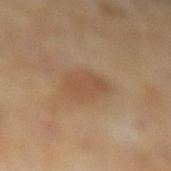Assessment: Imaged during a routine full-body skin examination; the lesion was not biopsied and no histopathology is available. Context: A region of skin cropped from a whole-body photographic capture, roughly 15 mm wide. The lesion is located on the left lower leg. A male patient aged 63 to 67.